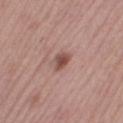The lesion was photographed on a routine skin check and not biopsied; there is no pathology result.
Approximately 2.5 mm at its widest.
A female patient aged approximately 40.
A 15 mm close-up tile from a total-body photography series done for melanoma screening.
The lesion is on the right thigh.
Imaged with white-light lighting.